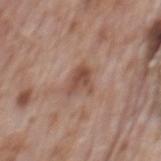Q: What did automated image analysis measure?
A: an outline eccentricity of about 0.5 (0 = round, 1 = elongated) and a shape-asymmetry score of about 0.45 (0 = symmetric); a mean CIELAB color near L≈49 a*≈20 b*≈27, about 10 CIELAB-L* units darker than the surrounding skin, and a lesion-to-skin contrast of about 7.5 (normalized; higher = more distinct); a border-irregularity rating of about 4.5/10 and internal color variation of about 3.5 on a 0–10 scale
Q: Who is the patient?
A: male, in their 70s
Q: Where on the body is the lesion?
A: the mid back
Q: What is the lesion's diameter?
A: ~3 mm (longest diameter)
Q: What is the imaging modality?
A: ~15 mm crop, total-body skin-cancer survey
Q: Illumination type?
A: white-light illumination